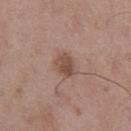  image:
    source: total-body photography crop
    field_of_view_mm: 15
  automated_metrics:
    cielab_L: 48
    cielab_a: 19
    cielab_b: 25
    vs_skin_contrast_norm: 7.5
    border_irregularity_0_10: 2.0
    color_variation_0_10: 2.5
    peripheral_color_asymmetry: 1.0
    nevus_likeness_0_100: 65
    lesion_detection_confidence_0_100: 100
  lighting: white-light
  patient:
    sex: male
    age_approx: 50
  site: left thigh